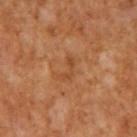Imaged during a routine full-body skin examination; the lesion was not biopsied and no histopathology is available. Imaged with cross-polarized lighting. The subject is a male roughly 65 years of age. A close-up tile cropped from a whole-body skin photograph, about 15 mm across. Longest diameter approximately 3 mm. The lesion-visualizer software estimated an average lesion color of about L≈46 a*≈24 b*≈38 (CIELAB) and a lesion-to-skin contrast of about 5.5 (normalized; higher = more distinct).